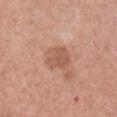| feature | finding |
|---|---|
| workup | catalogued during a skin exam; not biopsied |
| illumination | white-light illumination |
| automated lesion analysis | a nevus-likeness score of about 0/100 |
| lesion size | ~3 mm (longest diameter) |
| acquisition | ~15 mm crop, total-body skin-cancer survey |
| anatomic site | the right upper arm |
| subject | male, aged 53 to 57 |